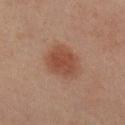No biopsy was performed on this lesion — it was imaged during a full skin examination and was not determined to be concerning. The patient is a female roughly 70 years of age. Cropped from a total-body skin-imaging series; the visible field is about 15 mm. From the right lower leg.